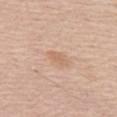| feature | finding |
|---|---|
| notes | catalogued during a skin exam; not biopsied |
| automated lesion analysis | an average lesion color of about L≈64 a*≈20 b*≈32 (CIELAB), roughly 7 lightness units darker than nearby skin, and a lesion-to-skin contrast of about 5 (normalized; higher = more distinct); a nevus-likeness score of about 0/100 and a lesion-detection confidence of about 100/100 |
| acquisition | ~15 mm tile from a whole-body skin photo |
| patient | female, aged 58–62 |
| diameter | about 2.5 mm |
| lighting | white-light illumination |
| site | the left thigh |